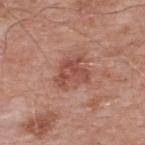Q: Was a biopsy performed?
A: total-body-photography surveillance lesion; no biopsy
Q: Illumination type?
A: white-light
Q: What are the patient's age and sex?
A: male, about 55 years old
Q: Automated lesion metrics?
A: a border-irregularity rating of about 5/10, a color-variation rating of about 3.5/10, and a peripheral color-asymmetry measure near 1.5; a lesion-detection confidence of about 100/100
Q: How was this image acquired?
A: total-body-photography crop, ~15 mm field of view
Q: Lesion location?
A: the back